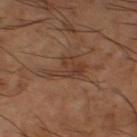Q: Was this lesion biopsied?
A: catalogued during a skin exam; not biopsied
Q: What is the lesion's diameter?
A: ~5.5 mm (longest diameter)
Q: What kind of image is this?
A: ~15 mm tile from a whole-body skin photo
Q: Lesion location?
A: the right thigh
Q: What are the patient's age and sex?
A: male, roughly 65 years of age
Q: Illumination type?
A: cross-polarized